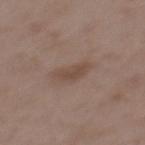Impression:
No biopsy was performed on this lesion — it was imaged during a full skin examination and was not determined to be concerning.
Background:
Imaged with white-light lighting. Automated tile analysis of the lesion measured a lesion color around L≈46 a*≈16 b*≈24 in CIELAB, a lesion–skin lightness drop of about 7, and a normalized border contrast of about 6. The software also gave a nevus-likeness score of about 0/100 and a detector confidence of about 100 out of 100 that the crop contains a lesion. A 15 mm crop from a total-body photograph taken for skin-cancer surveillance. Located on the mid back. A male subject roughly 50 years of age.